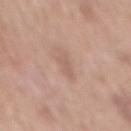| field | value |
|---|---|
| biopsy status | catalogued during a skin exam; not biopsied |
| lesion diameter | about 2.5 mm |
| tile lighting | white-light |
| site | the mid back |
| subject | male, in their 70s |
| image | ~15 mm tile from a whole-body skin photo |